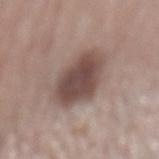Clinical impression: Captured during whole-body skin photography for melanoma surveillance; the lesion was not biopsied. Background: A region of skin cropped from a whole-body photographic capture, roughly 15 mm wide. A male patient approximately 65 years of age. Approximately 5.5 mm at its widest. The tile uses white-light illumination. The lesion is on the mid back.